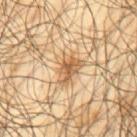Captured during whole-body skin photography for melanoma surveillance; the lesion was not biopsied.
Located on the upper back.
About 4 mm across.
The subject is a male in their mid-60s.
A region of skin cropped from a whole-body photographic capture, roughly 15 mm wide.
An algorithmic analysis of the crop reported border irregularity of about 4 on a 0–10 scale and radial color variation of about 1.5. The analysis additionally found a nevus-likeness score of about 65/100 and a lesion-detection confidence of about 95/100.